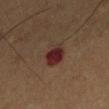{
  "biopsy_status": "not biopsied; imaged during a skin examination",
  "automated_metrics": {
    "area_mm2_approx": 7.0,
    "shape_asymmetry": 0.15,
    "cielab_L": 24,
    "cielab_a": 20,
    "cielab_b": 19,
    "border_irregularity_0_10": 1.5,
    "color_variation_0_10": 5.5,
    "peripheral_color_asymmetry": 2.0
  },
  "lesion_size": {
    "long_diameter_mm_approx": 3.0
  },
  "site": "left lower leg",
  "image": {
    "source": "total-body photography crop",
    "field_of_view_mm": 15
  },
  "patient": {
    "sex": "male",
    "age_approx": 55
  },
  "lighting": "cross-polarized"
}